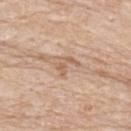Impression: Captured during whole-body skin photography for melanoma surveillance; the lesion was not biopsied. Clinical summary: A male patient approximately 85 years of age or older. Imaged with white-light lighting. On the upper back. This image is a 15 mm lesion crop taken from a total-body photograph. About 3 mm across.